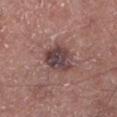follow-up = total-body-photography surveillance lesion; no biopsy
illumination = white-light illumination
automated lesion analysis = a border-irregularity index near 2/10 and a peripheral color-asymmetry measure near 1.5; an automated nevus-likeness rating near 25 out of 100
size = ≈4 mm
patient = male, in their mid-50s
location = the left lower leg
image = ~15 mm tile from a whole-body skin photo